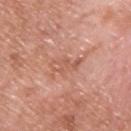Clinical impression:
The lesion was photographed on a routine skin check and not biopsied; there is no pathology result.
Clinical summary:
About 3.5 mm across. A 15 mm crop from a total-body photograph taken for skin-cancer surveillance. The total-body-photography lesion software estimated roughly 7 lightness units darker than nearby skin and a normalized border contrast of about 5. And it measured a nevus-likeness score of about 0/100 and a detector confidence of about 100 out of 100 that the crop contains a lesion. Captured under white-light illumination. On the back. A male patient, aged around 55.